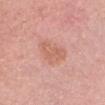The lesion was photographed on a routine skin check and not biopsied; there is no pathology result. A 15 mm close-up extracted from a 3D total-body photography capture. On the head or neck. The subject is a female aged 28–32. The tile uses white-light illumination.